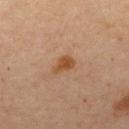Impression: This lesion was catalogued during total-body skin photography and was not selected for biopsy. Acquisition and patient details: A female patient, in their mid-40s. A 15 mm crop from a total-body photograph taken for skin-cancer surveillance. An algorithmic analysis of the crop reported a classifier nevus-likeness of about 70/100 and a lesion-detection confidence of about 100/100. Captured under cross-polarized illumination. The recorded lesion diameter is about 3 mm. From the upper back.